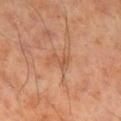Imaged during a routine full-body skin examination; the lesion was not biopsied and no histopathology is available. The lesion is located on the leg. A 15 mm close-up tile from a total-body photography series done for melanoma screening. The patient is a male aged 68 to 72. The total-body-photography lesion software estimated lesion-presence confidence of about 95/100.